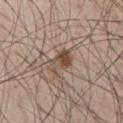This image is a 15 mm lesion crop taken from a total-body photograph.
The tile uses white-light illumination.
A male patient, aged 48–52.
On the chest.